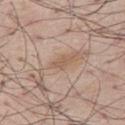The lesion was tiled from a total-body skin photograph and was not biopsied. Located on the left leg. Cropped from a whole-body photographic skin survey; the tile spans about 15 mm. A male patient, aged 68–72.